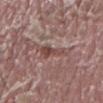biopsy status: no biopsy performed (imaged during a skin exam)
lighting: white-light illumination
patient: male, aged 38 to 42
size: ~3.5 mm (longest diameter)
location: the back
automated metrics: an average lesion color of about L≈46 a*≈21 b*≈21 (CIELAB), about 9 CIELAB-L* units darker than the surrounding skin, and a lesion-to-skin contrast of about 7 (normalized; higher = more distinct)
acquisition: ~15 mm crop, total-body skin-cancer survey